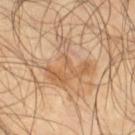Assessment: This lesion was catalogued during total-body skin photography and was not selected for biopsy. Background: An algorithmic analysis of the crop reported a shape eccentricity near 0.45 and a symmetry-axis asymmetry near 0.7. And it measured a mean CIELAB color near L≈54 a*≈18 b*≈33, a lesion–skin lightness drop of about 7, and a normalized border contrast of about 5.5. It also reported a border-irregularity rating of about 10/10, a within-lesion color-variation index near 3/10, and radial color variation of about 0.5. Longest diameter approximately 5 mm. This is a cross-polarized tile. On the right thigh. A male patient, aged 53–57. Cropped from a total-body skin-imaging series; the visible field is about 15 mm.